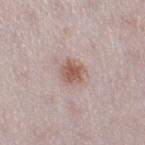biopsy status = imaged on a skin check; not biopsied | anatomic site = the right thigh | lesion size = ≈3 mm | image source = ~15 mm tile from a whole-body skin photo | subject = female, approximately 30 years of age.